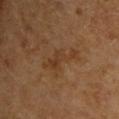– biopsy status — total-body-photography surveillance lesion; no biopsy
– automated lesion analysis — a footprint of about 8 mm², an outline eccentricity of about 0.9 (0 = round, 1 = elongated), and a shape-asymmetry score of about 0.45 (0 = symmetric); a classifier nevus-likeness of about 0/100
– body site — the upper back
– image source — ~15 mm crop, total-body skin-cancer survey
– tile lighting — cross-polarized
– patient — male, about 65 years old